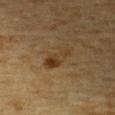lesion size = about 4 mm | tile lighting = cross-polarized illumination | imaging modality = ~15 mm tile from a whole-body skin photo | body site = the chest | automated lesion analysis = a footprint of about 6.5 mm², an eccentricity of roughly 0.9, and a symmetry-axis asymmetry near 0.3; a border-irregularity rating of about 3.5/10, a within-lesion color-variation index near 6.5/10, and radial color variation of about 2.5; a classifier nevus-likeness of about 75/100 and a lesion-detection confidence of about 100/100 | patient = male, aged around 85 or older.